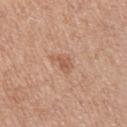Case summary:
• notes — total-body-photography surveillance lesion; no biopsy
• patient — female, aged around 50
• tile lighting — white-light
• lesion diameter — about 2.5 mm
• image — 15 mm crop, total-body photography
• anatomic site — the right upper arm